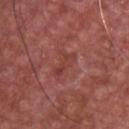biopsy status — catalogued during a skin exam; not biopsied
subject — male, approximately 65 years of age
body site — the front of the torso
lesion size — ≈3.5 mm
image — 15 mm crop, total-body photography
tile lighting — white-light
automated metrics — an area of roughly 4 mm², an eccentricity of roughly 0.9, and a shape-asymmetry score of about 0.4 (0 = symmetric); a lesion color around L≈41 a*≈28 b*≈26 in CIELAB, roughly 6 lightness units darker than nearby skin, and a lesion-to-skin contrast of about 5.5 (normalized; higher = more distinct); a border-irregularity rating of about 5/10, a within-lesion color-variation index near 0/10, and radial color variation of about 0; a nevus-likeness score of about 0/100 and a detector confidence of about 100 out of 100 that the crop contains a lesion